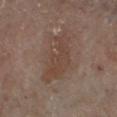• tile lighting — cross-polarized illumination
• subject — female, roughly 60 years of age
• body site — the leg
• image — 15 mm crop, total-body photography